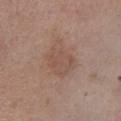Q: Was this lesion biopsied?
A: no biopsy performed (imaged during a skin exam)
Q: Where on the body is the lesion?
A: the left lower leg
Q: What is the imaging modality?
A: 15 mm crop, total-body photography
Q: Who is the patient?
A: male, aged approximately 55